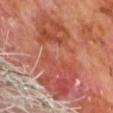From the back.
A male subject, aged 58 to 62.
Cropped from a whole-body photographic skin survey; the tile spans about 15 mm.